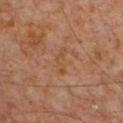Notes:
* biopsy status — total-body-photography surveillance lesion; no biopsy
* imaging modality — ~15 mm tile from a whole-body skin photo
* diameter — about 3.5 mm
* subject — male, in their 60s
* location — the upper back
* tile lighting — cross-polarized
* TBP lesion metrics — an area of roughly 4 mm², an eccentricity of roughly 0.9, and two-axis asymmetry of about 0.6; an average lesion color of about L≈43 a*≈19 b*≈31 (CIELAB), about 4 CIELAB-L* units darker than the surrounding skin, and a lesion-to-skin contrast of about 5 (normalized; higher = more distinct); a border-irregularity index near 6.5/10, a color-variation rating of about 1/10, and a peripheral color-asymmetry measure near 0